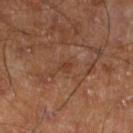{
  "biopsy_status": "not biopsied; imaged during a skin examination",
  "site": "left lower leg",
  "automated_metrics": {
    "nevus_likeness_0_100": 0
  },
  "image": {
    "source": "total-body photography crop",
    "field_of_view_mm": 15
  },
  "patient": {
    "sex": "male",
    "age_approx": 60
  }
}